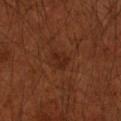Part of a total-body skin-imaging series; this lesion was reviewed on a skin check and was not flagged for biopsy.
The lesion-visualizer software estimated a lesion area of about 4 mm², a shape eccentricity near 0.65, and two-axis asymmetry of about 0.35. The software also gave radial color variation of about 0.5.
Captured under cross-polarized illumination.
Approximately 2.5 mm at its widest.
From the left arm.
A male patient, aged around 50.
Cropped from a whole-body photographic skin survey; the tile spans about 15 mm.